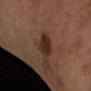The lesion was photographed on a routine skin check and not biopsied; there is no pathology result. Automated image analysis of the tile measured border irregularity of about 2 on a 0–10 scale, internal color variation of about 2.5 on a 0–10 scale, and a peripheral color-asymmetry measure near 1. The subject is a male aged 63 to 67. A 15 mm close-up extracted from a 3D total-body photography capture. The lesion's longest dimension is about 3 mm.